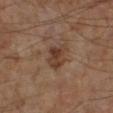The lesion was photographed on a routine skin check and not biopsied; there is no pathology result. On the left lower leg. The lesion's longest dimension is about 3.5 mm. A lesion tile, about 15 mm wide, cut from a 3D total-body photograph. Captured under cross-polarized illumination. A male subject, approximately 60 years of age. An algorithmic analysis of the crop reported an area of roughly 6.5 mm², an eccentricity of roughly 0.7, and a symmetry-axis asymmetry near 0.3. The analysis additionally found a lesion color around L≈37 a*≈17 b*≈26 in CIELAB, a lesion–skin lightness drop of about 9, and a lesion-to-skin contrast of about 8 (normalized; higher = more distinct).